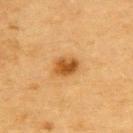* automated metrics: an outline eccentricity of about 0.7 (0 = round, 1 = elongated) and two-axis asymmetry of about 0.2; a lesion–skin lightness drop of about 12
* image: ~15 mm tile from a whole-body skin photo
* tile lighting: cross-polarized illumination
* patient: male, approximately 85 years of age or older
* size: about 3 mm
* location: the upper back